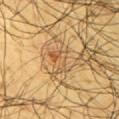Clinical impression:
This lesion was catalogued during total-body skin photography and was not selected for biopsy.
Clinical summary:
A male subject, in their mid- to late 60s. A 15 mm crop from a total-body photograph taken for skin-cancer surveillance. The total-body-photography lesion software estimated a footprint of about 4.5 mm² and a shape-asymmetry score of about 0.6 (0 = symmetric). The software also gave a lesion–skin lightness drop of about 9 and a lesion-to-skin contrast of about 6 (normalized; higher = more distinct). And it measured an automated nevus-likeness rating near 15 out of 100 and a lesion-detection confidence of about 75/100. The tile uses cross-polarized illumination. The lesion is on the right upper arm. Approximately 3 mm at its widest.